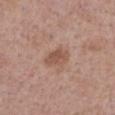<record>
  <biopsy_status>not biopsied; imaged during a skin examination</biopsy_status>
  <site>right forearm</site>
  <patient>
    <sex>female</sex>
    <age_approx>40</age_approx>
  </patient>
  <image>
    <source>total-body photography crop</source>
    <field_of_view_mm>15</field_of_view_mm>
  </image>
  <lighting>white-light</lighting>
  <lesion_size>
    <long_diameter_mm_approx>3.0</long_diameter_mm_approx>
  </lesion_size>
</record>